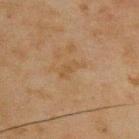| field | value |
|---|---|
| biopsy status | total-body-photography surveillance lesion; no biopsy |
| tile lighting | cross-polarized illumination |
| lesion diameter | ≈3 mm |
| image-analysis metrics | an area of roughly 4 mm², an outline eccentricity of about 0.85 (0 = round, 1 = elongated), and a symmetry-axis asymmetry near 0.4; a border-irregularity index near 4.5/10, a within-lesion color-variation index near 1.5/10, and radial color variation of about 0.5; lesion-presence confidence of about 100/100 |
| anatomic site | the back |
| acquisition | ~15 mm crop, total-body skin-cancer survey |
| patient | male, aged approximately 45 |